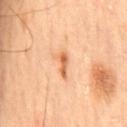No biopsy was performed on this lesion — it was imaged during a full skin examination and was not determined to be concerning. The total-body-photography lesion software estimated a shape-asymmetry score of about 0.5 (0 = symmetric). The analysis additionally found an average lesion color of about L≈64 a*≈27 b*≈42 (CIELAB), a lesion–skin lightness drop of about 13, and a normalized lesion–skin contrast near 8.5. The analysis additionally found a classifier nevus-likeness of about 90/100 and lesion-presence confidence of about 100/100. Captured under cross-polarized illumination. A male subject in their mid-60s. Measured at roughly 3 mm in maximum diameter. A close-up tile cropped from a whole-body skin photograph, about 15 mm across. Located on the chest.